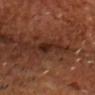  biopsy_status: not biopsied; imaged during a skin examination
  lighting: cross-polarized
  patient:
    sex: male
    age_approx: 55
  automated_metrics:
    area_mm2_approx: 4.5
    eccentricity: 0.75
    shape_asymmetry: 0.35
    cielab_L: 23
    cielab_a: 19
    cielab_b: 23
    vs_skin_darker_L: 7.0
    vs_skin_contrast_norm: 8.0
    nevus_likeness_0_100: 5
    lesion_detection_confidence_0_100: 100
  site: head or neck
  image:
    source: total-body photography crop
    field_of_view_mm: 15